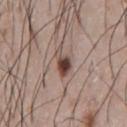biopsy status — no biopsy performed (imaged during a skin exam)
patient — male, in their mid- to late 70s
automated metrics — a footprint of about 5 mm², an outline eccentricity of about 0.8 (0 = round, 1 = elongated), and a shape-asymmetry score of about 0.35 (0 = symmetric); border irregularity of about 3.5 on a 0–10 scale and internal color variation of about 6.5 on a 0–10 scale; a classifier nevus-likeness of about 100/100
site — the chest
size — ≈3.5 mm
imaging modality — ~15 mm crop, total-body skin-cancer survey